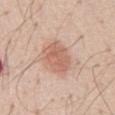{
  "biopsy_status": "not biopsied; imaged during a skin examination",
  "image": {
    "source": "total-body photography crop",
    "field_of_view_mm": 15
  },
  "patient": {
    "sex": "male",
    "age_approx": 70
  },
  "site": "abdomen"
}